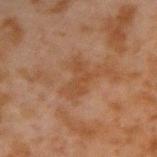Captured during whole-body skin photography for melanoma surveillance; the lesion was not biopsied. This image is a 15 mm lesion crop taken from a total-body photograph. The lesion's longest dimension is about 4 mm. From the right upper arm. The patient is a male in their mid-40s.